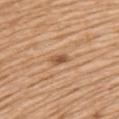Context: Cropped from a total-body skin-imaging series; the visible field is about 15 mm. On the back. A male subject, about 70 years old. About 2.5 mm across.